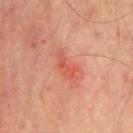Notes:
• biopsy status: no biopsy performed (imaged during a skin exam)
• anatomic site: the chest
• subject: male, in their mid-60s
• imaging modality: total-body-photography crop, ~15 mm field of view